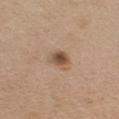notes=no biopsy performed (imaged during a skin exam)
site=the chest
image=~15 mm tile from a whole-body skin photo
lesion size=≈2.5 mm
lighting=white-light illumination
subject=female, aged 43–47